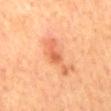Recorded during total-body skin imaging; not selected for excision or biopsy. The patient is a male aged around 70. Longest diameter approximately 5 mm. Located on the mid back. Cropped from a total-body skin-imaging series; the visible field is about 15 mm. The tile uses cross-polarized illumination.